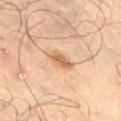Notes:
- notes · total-body-photography surveillance lesion; no biopsy
- patient · male, in their 70s
- site · the right thigh
- imaging modality · total-body-photography crop, ~15 mm field of view